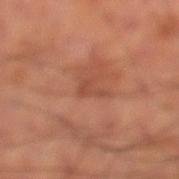Part of a total-body skin-imaging series; this lesion was reviewed on a skin check and was not flagged for biopsy. Longest diameter approximately 2.5 mm. This image is a 15 mm lesion crop taken from a total-body photograph. A male patient about 65 years old. The lesion-visualizer software estimated an area of roughly 2 mm² and an eccentricity of roughly 0.85. The analysis additionally found a border-irregularity rating of about 8/10 and radial color variation of about 0. The software also gave a lesion-detection confidence of about 90/100. Imaged with cross-polarized lighting. The lesion is on the left lower leg.